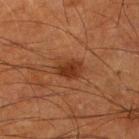biopsy status: total-body-photography surveillance lesion; no biopsy
automated metrics: a lesion area of about 5.5 mm², an eccentricity of roughly 0.75, and a shape-asymmetry score of about 0.25 (0 = symmetric); a border-irregularity rating of about 2/10 and a within-lesion color-variation index near 2.5/10; an automated nevus-likeness rating near 95 out of 100
size: ~3.5 mm (longest diameter)
subject: male, aged approximately 65
body site: the right lower leg
imaging modality: ~15 mm crop, total-body skin-cancer survey